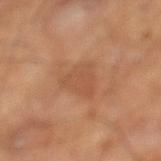  biopsy_status: not biopsied; imaged during a skin examination
  site: leg
  lesion_size:
    long_diameter_mm_approx: 4.5
  image:
    source: total-body photography crop
    field_of_view_mm: 15
  automated_metrics:
    nevus_likeness_0_100: 0
    lesion_detection_confidence_0_100: 100
  patient:
    sex: male
    age_approx: 65
  lighting: cross-polarized